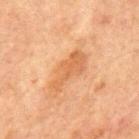biopsy_status: not biopsied; imaged during a skin examination
lesion_size:
  long_diameter_mm_approx: 5.5
site: chest
image:
  source: total-body photography crop
  field_of_view_mm: 15
patient:
  sex: male
  age_approx: 65
automated_metrics:
  eccentricity: 0.9
  shape_asymmetry: 0.4
  border_irregularity_0_10: 4.5
  color_variation_0_10: 2.0